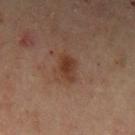notes — imaged on a skin check; not biopsied | lighting — cross-polarized | location — the left upper arm | patient — male, about 45 years old | acquisition — total-body-photography crop, ~15 mm field of view.